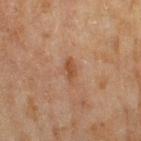Imaged during a routine full-body skin examination; the lesion was not biopsied and no histopathology is available. Captured under cross-polarized illumination. A female subject, aged 58 to 62. Located on the right thigh. A 15 mm close-up extracted from a 3D total-body photography capture.